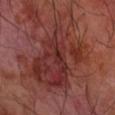Part of a total-body skin-imaging series; this lesion was reviewed on a skin check and was not flagged for biopsy. A 15 mm close-up tile from a total-body photography series done for melanoma screening. Imaged with cross-polarized lighting. Longest diameter approximately 11 mm. A male patient aged around 70. Located on the right forearm.